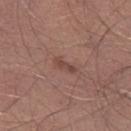Part of a total-body skin-imaging series; this lesion was reviewed on a skin check and was not flagged for biopsy.
The total-body-photography lesion software estimated border irregularity of about 3.5 on a 0–10 scale, a within-lesion color-variation index near 0/10, and radial color variation of about 0. It also reported a nevus-likeness score of about 5/100 and lesion-presence confidence of about 100/100.
This is a white-light tile.
Approximately 3 mm at its widest.
The lesion is on the right thigh.
The subject is a male in their mid-20s.
This image is a 15 mm lesion crop taken from a total-body photograph.